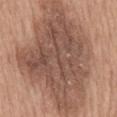Findings:
• imaging modality: ~15 mm crop, total-body skin-cancer survey
• lighting: white-light
• body site: the mid back
• automated lesion analysis: an outline eccentricity of about 0.65 (0 = round, 1 = elongated) and a shape-asymmetry score of about 0.25 (0 = symmetric); a lesion–skin lightness drop of about 12; border irregularity of about 3.5 on a 0–10 scale, a within-lesion color-variation index near 5.5/10, and a peripheral color-asymmetry measure near 2
• subject: male, aged around 60